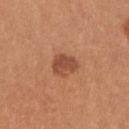biopsy_status: not biopsied; imaged during a skin examination
automated_metrics:
  area_mm2_approx: 6.0
  eccentricity: 0.55
  shape_asymmetry: 0.25
  vs_skin_darker_L: 10.0
  color_variation_0_10: 2.5
  peripheral_color_asymmetry: 1.0
lesion_size:
  long_diameter_mm_approx: 3.0
patient:
  sex: female
  age_approx: 25
image:
  source: total-body photography crop
  field_of_view_mm: 15
site: right upper arm